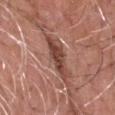  biopsy_status: not biopsied; imaged during a skin examination
  lighting: white-light
  patient:
    sex: male
    age_approx: 60
  site: head or neck
  automated_metrics:
    area_mm2_approx: 8.5
    eccentricity: 0.95
    shape_asymmetry: 0.25
    cielab_L: 44
    cielab_a: 23
    cielab_b: 26
    vs_skin_darker_L: 12.0
    color_variation_0_10: 3.0
    peripheral_color_asymmetry: 1.0
    nevus_likeness_0_100: 20
    lesion_detection_confidence_0_100: 95
  image:
    source: total-body photography crop
    field_of_view_mm: 15